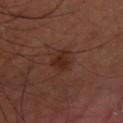notes: total-body-photography surveillance lesion; no biopsy
patient: male, in their 50s
acquisition: total-body-photography crop, ~15 mm field of view
TBP lesion metrics: an area of roughly 6 mm², an eccentricity of roughly 0.55, and a shape-asymmetry score of about 0.25 (0 = symmetric)
lighting: cross-polarized
anatomic site: the left arm
diameter: ≈3 mm